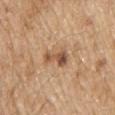Q: Was this lesion biopsied?
A: total-body-photography surveillance lesion; no biopsy
Q: Automated lesion metrics?
A: a peripheral color-asymmetry measure near 1; a lesion-detection confidence of about 100/100
Q: Where on the body is the lesion?
A: the upper back
Q: Lesion size?
A: about 3.5 mm
Q: What is the imaging modality?
A: ~15 mm tile from a whole-body skin photo
Q: What lighting was used for the tile?
A: white-light illumination
Q: Patient demographics?
A: male, aged around 70